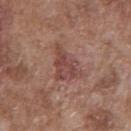Clinical impression: This lesion was catalogued during total-body skin photography and was not selected for biopsy. Context: This image is a 15 mm lesion crop taken from a total-body photograph. Captured under white-light illumination. On the chest. A male patient aged 73 to 77. Measured at roughly 4.5 mm in maximum diameter.